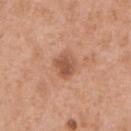Clinical impression:
Imaged during a routine full-body skin examination; the lesion was not biopsied and no histopathology is available.
Acquisition and patient details:
Automated image analysis of the tile measured a lesion color around L≈54 a*≈24 b*≈32 in CIELAB, roughly 11 lightness units darker than nearby skin, and a normalized border contrast of about 7.5. It also reported a nevus-likeness score of about 20/100. A region of skin cropped from a whole-body photographic capture, roughly 15 mm wide. Located on the chest. A male subject, aged approximately 55.